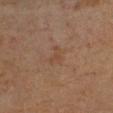biopsy status: total-body-photography surveillance lesion; no biopsy | lesion size: ≈2.5 mm | tile lighting: cross-polarized | location: the left forearm | image source: total-body-photography crop, ~15 mm field of view | patient: female, aged 63 to 67 | image-analysis metrics: an average lesion color of about L≈38 a*≈16 b*≈25 (CIELAB), roughly 4 lightness units darker than nearby skin, and a normalized lesion–skin contrast near 4.5; a nevus-likeness score of about 0/100.